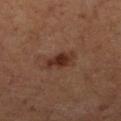Findings:
• follow-up · total-body-photography surveillance lesion; no biopsy
• acquisition · ~15 mm crop, total-body skin-cancer survey
• patient · female
• body site · the right lower leg
• tile lighting · cross-polarized
• image-analysis metrics · an average lesion color of about L≈32 a*≈21 b*≈27 (CIELAB), roughly 10 lightness units darker than nearby skin, and a normalized border contrast of about 9; a border-irregularity index near 2.5/10, a color-variation rating of about 2.5/10, and a peripheral color-asymmetry measure near 0.5; a nevus-likeness score of about 80/100 and a lesion-detection confidence of about 100/100